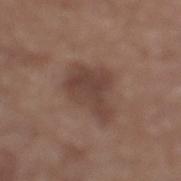Q: Was a biopsy performed?
A: imaged on a skin check; not biopsied
Q: Lesion size?
A: about 5.5 mm
Q: Illumination type?
A: white-light
Q: How was this image acquired?
A: total-body-photography crop, ~15 mm field of view
Q: Patient demographics?
A: male, approximately 65 years of age
Q: Automated lesion metrics?
A: a lesion area of about 14 mm², a shape eccentricity near 0.75, and a shape-asymmetry score of about 0.35 (0 = symmetric); an automated nevus-likeness rating near 25 out of 100 and a lesion-detection confidence of about 100/100
Q: Where on the body is the lesion?
A: the left lower leg